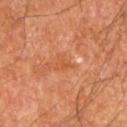A 15 mm close-up extracted from a 3D total-body photography capture.
Located on the right upper arm.
The total-body-photography lesion software estimated a lesion area of about 3 mm², a shape eccentricity near 0.7, and a shape-asymmetry score of about 0.55 (0 = symmetric). The analysis additionally found a border-irregularity rating of about 5.5/10. And it measured an automated nevus-likeness rating near 0 out of 100 and a lesion-detection confidence of about 100/100.
This is a cross-polarized tile.
A male subject, about 65 years old.